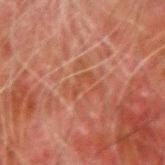No biopsy was performed on this lesion — it was imaged during a full skin examination and was not determined to be concerning.
A male patient about 80 years old.
An algorithmic analysis of the crop reported a border-irregularity rating of about 6/10, internal color variation of about 0 on a 0–10 scale, and peripheral color asymmetry of about 0.
Longest diameter approximately 3 mm.
On the left forearm.
A 15 mm close-up tile from a total-body photography series done for melanoma screening.